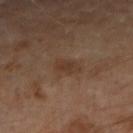<record>
  <biopsy_status>not biopsied; imaged during a skin examination</biopsy_status>
  <site>right forearm</site>
  <lighting>cross-polarized</lighting>
  <lesion_size>
    <long_diameter_mm_approx>3.0</long_diameter_mm_approx>
  </lesion_size>
  <patient>
    <sex>female</sex>
    <age_approx>60</age_approx>
  </patient>
  <image>
    <source>total-body photography crop</source>
    <field_of_view_mm>15</field_of_view_mm>
  </image>
  <automated_metrics>
    <cielab_L>36</cielab_L>
    <cielab_a>17</cielab_a>
    <cielab_b>27</cielab_b>
    <vs_skin_darker_L>7.0</vs_skin_darker_L>
    <vs_skin_contrast_norm>6.0</vs_skin_contrast_norm>
    <border_irregularity_0_10>2.0</border_irregularity_0_10>
    <color_variation_0_10>1.0</color_variation_0_10>
    <peripheral_color_asymmetry>0.5</peripheral_color_asymmetry>
    <nevus_likeness_0_100>0</nevus_likeness_0_100>
  </automated_metrics>
</record>